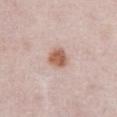Notes:
– biopsy status — total-body-photography surveillance lesion; no biopsy
– anatomic site — the abdomen
– imaging modality — total-body-photography crop, ~15 mm field of view
– patient — female, about 50 years old